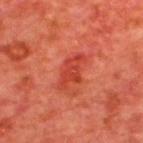Recorded during total-body skin imaging; not selected for excision or biopsy.
A 15 mm close-up extracted from a 3D total-body photography capture.
The lesion is located on the upper back.
The total-body-photography lesion software estimated an eccentricity of roughly 0.9. The analysis additionally found a mean CIELAB color near L≈41 a*≈38 b*≈34, a lesion–skin lightness drop of about 8, and a lesion-to-skin contrast of about 6.5 (normalized; higher = more distinct). The analysis additionally found a border-irregularity index near 4.5/10, a color-variation rating of about 2/10, and a peripheral color-asymmetry measure near 0.5. The software also gave a nevus-likeness score of about 60/100 and a lesion-detection confidence of about 100/100.
Captured under cross-polarized illumination.
A male patient, aged approximately 65.
Measured at roughly 4.5 mm in maximum diameter.